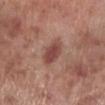Imaged during a routine full-body skin examination; the lesion was not biopsied and no histopathology is available.